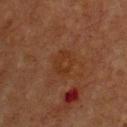No biopsy was performed on this lesion — it was imaged during a full skin examination and was not determined to be concerning.
The subject is a male aged approximately 75.
The tile uses cross-polarized illumination.
This image is a 15 mm lesion crop taken from a total-body photograph.
The lesion is located on the chest.